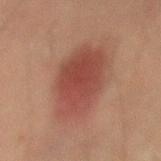Imaged during a routine full-body skin examination; the lesion was not biopsied and no histopathology is available. A male patient approximately 45 years of age. Imaged with cross-polarized lighting. From the mid back. A 15 mm close-up extracted from a 3D total-body photography capture. Automated image analysis of the tile measured a footprint of about 26 mm². The software also gave a lesion color around L≈37 a*≈22 b*≈23 in CIELAB, about 9 CIELAB-L* units darker than the surrounding skin, and a normalized lesion–skin contrast near 8.